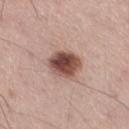Clinical impression: Captured during whole-body skin photography for melanoma surveillance; the lesion was not biopsied. Context: A lesion tile, about 15 mm wide, cut from a 3D total-body photograph. The lesion is located on the leg. A male patient aged 53–57.